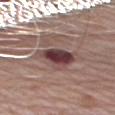Q: Was this lesion biopsied?
A: catalogued during a skin exam; not biopsied
Q: Lesion location?
A: the arm
Q: What lighting was used for the tile?
A: white-light illumination
Q: Lesion size?
A: about 6 mm
Q: Automated lesion metrics?
A: a lesion area of about 12 mm², an eccentricity of roughly 0.85, and two-axis asymmetry of about 0.35
Q: Who is the patient?
A: male, aged around 70
Q: What kind of image is this?
A: ~15 mm tile from a whole-body skin photo